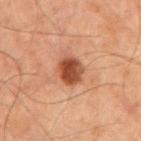| field | value |
|---|---|
| follow-up | total-body-photography surveillance lesion; no biopsy |
| diameter | ≈3 mm |
| image-analysis metrics | a footprint of about 7 mm², an outline eccentricity of about 0.45 (0 = round, 1 = elongated), and a symmetry-axis asymmetry near 0.1; a border-irregularity index near 1/10, internal color variation of about 3.5 on a 0–10 scale, and a peripheral color-asymmetry measure near 1; an automated nevus-likeness rating near 100 out of 100 |
| lighting | cross-polarized illumination |
| acquisition | ~15 mm crop, total-body skin-cancer survey |
| subject | male, aged 68 to 72 |
| anatomic site | the chest |